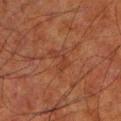workup: imaged on a skin check; not biopsied
site: the left lower leg
image source: ~15 mm tile from a whole-body skin photo
lesion diameter: ≈3 mm
tile lighting: cross-polarized
subject: male, aged 78–82
TBP lesion metrics: a border-irregularity rating of about 8/10, a within-lesion color-variation index near 0.5/10, and a peripheral color-asymmetry measure near 0